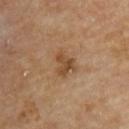Case summary:
* notes — total-body-photography surveillance lesion; no biopsy
* imaging modality — ~15 mm crop, total-body skin-cancer survey
* lesion size — ≈3 mm
* illumination — cross-polarized illumination
* subject — male, in their mid- to late 80s or older
* location — the upper back